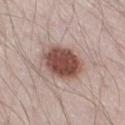Q: Was this lesion biopsied?
A: no biopsy performed (imaged during a skin exam)
Q: What is the imaging modality?
A: ~15 mm crop, total-body skin-cancer survey
Q: Illumination type?
A: white-light illumination
Q: Who is the patient?
A: male, aged 38–42
Q: How large is the lesion?
A: ≈5 mm
Q: Lesion location?
A: the right thigh